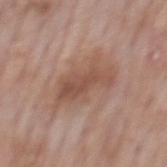Captured during whole-body skin photography for melanoma surveillance; the lesion was not biopsied. A male patient aged approximately 75. A close-up tile cropped from a whole-body skin photograph, about 15 mm across. The lesion is on the mid back.